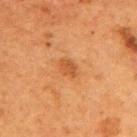Q: Is there a histopathology result?
A: catalogued during a skin exam; not biopsied
Q: What is the anatomic site?
A: the mid back
Q: What is the lesion's diameter?
A: ~2.5 mm (longest diameter)
Q: How was the tile lit?
A: cross-polarized
Q: Automated lesion metrics?
A: a footprint of about 3 mm² and a shape eccentricity near 0.75; an average lesion color of about L≈44 a*≈24 b*≈37 (CIELAB) and about 8 CIELAB-L* units darker than the surrounding skin; a border-irregularity index near 3/10, a color-variation rating of about 1.5/10, and a peripheral color-asymmetry measure near 0.5
Q: Patient demographics?
A: female, aged 48–52
Q: What kind of image is this?
A: ~15 mm tile from a whole-body skin photo